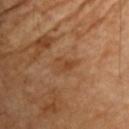The lesion was photographed on a routine skin check and not biopsied; there is no pathology result.